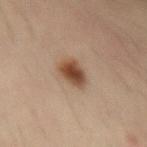{"biopsy_status": "not biopsied; imaged during a skin examination", "site": "left forearm", "lesion_size": {"long_diameter_mm_approx": 3.0}, "image": {"source": "total-body photography crop", "field_of_view_mm": 15}, "patient": {"sex": "male", "age_approx": 35}, "lighting": "cross-polarized"}